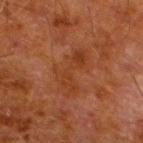{
  "biopsy_status": "not biopsied; imaged during a skin examination",
  "image": {
    "source": "total-body photography crop",
    "field_of_view_mm": 15
  },
  "lesion_size": {
    "long_diameter_mm_approx": 6.0
  },
  "lighting": "cross-polarized",
  "site": "left lower leg",
  "patient": {
    "sex": "male",
    "age_approx": 80
  },
  "automated_metrics": {
    "eccentricity": 0.85,
    "shape_asymmetry": 0.55,
    "lesion_detection_confidence_0_100": 100
  }
}